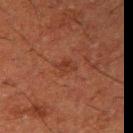The lesion was photographed on a routine skin check and not biopsied; there is no pathology result. The patient is a male aged 48 to 52. A roughly 15 mm field-of-view crop from a total-body skin photograph. The tile uses cross-polarized illumination. Located on the right upper arm. The lesion's longest dimension is about 2.5 mm.